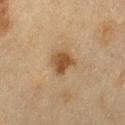Q: Was a biopsy performed?
A: catalogued during a skin exam; not biopsied
Q: Automated lesion metrics?
A: about 11 CIELAB-L* units darker than the surrounding skin; a classifier nevus-likeness of about 85/100 and a lesion-detection confidence of about 100/100
Q: What is the lesion's diameter?
A: ≈3 mm
Q: What kind of image is this?
A: ~15 mm crop, total-body skin-cancer survey
Q: Lesion location?
A: the left thigh
Q: What lighting was used for the tile?
A: cross-polarized illumination
Q: Patient demographics?
A: female, roughly 55 years of age